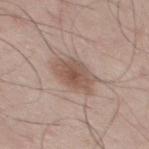Impression:
Part of a total-body skin-imaging series; this lesion was reviewed on a skin check and was not flagged for biopsy.
Clinical summary:
Measured at roughly 4.5 mm in maximum diameter. Cropped from a whole-body photographic skin survey; the tile spans about 15 mm. Automated image analysis of the tile measured a border-irregularity rating of about 2/10, a within-lesion color-variation index near 3.5/10, and radial color variation of about 1. Captured under white-light illumination. The patient is a male roughly 45 years of age. On the mid back.